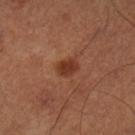Impression: Recorded during total-body skin imaging; not selected for excision or biopsy. Clinical summary: Longest diameter approximately 2.5 mm. The lesion-visualizer software estimated a lesion color around L≈36 a*≈25 b*≈31 in CIELAB and roughly 9 lightness units darker than nearby skin. The analysis additionally found border irregularity of about 1.5 on a 0–10 scale, a within-lesion color-variation index near 2/10, and radial color variation of about 0.5. It also reported a classifier nevus-likeness of about 95/100. This is a cross-polarized tile. Located on the leg. A 15 mm close-up extracted from a 3D total-body photography capture. A male patient about 50 years old.